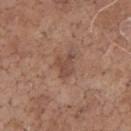The lesion was tiled from a total-body skin photograph and was not biopsied. The patient is a male about 70 years old. Cropped from a whole-body photographic skin survey; the tile spans about 15 mm. Approximately 3 mm at its widest. The lesion is on the chest. An algorithmic analysis of the crop reported a lesion area of about 5.5 mm² and an eccentricity of roughly 0.65. And it measured a mean CIELAB color near L≈47 a*≈20 b*≈26, a lesion–skin lightness drop of about 8, and a lesion-to-skin contrast of about 6 (normalized; higher = more distinct). The software also gave a border-irregularity rating of about 4/10, a within-lesion color-variation index near 1.5/10, and a peripheral color-asymmetry measure near 0.5. The analysis additionally found a nevus-likeness score of about 0/100 and a lesion-detection confidence of about 100/100.